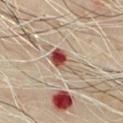Case summary:
• follow-up · no biopsy performed (imaged during a skin exam)
• diameter · ~4 mm (longest diameter)
• location · the chest
• subject · male, aged approximately 55
• image · 15 mm crop, total-body photography
• automated metrics · a footprint of about 6 mm², a shape eccentricity near 0.85, and a shape-asymmetry score of about 0.45 (0 = symmetric); a lesion color around L≈53 a*≈24 b*≈28 in CIELAB, about 16 CIELAB-L* units darker than the surrounding skin, and a normalized lesion–skin contrast near 11; an automated nevus-likeness rating near 0 out of 100 and a lesion-detection confidence of about 100/100